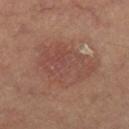The lesion was photographed on a routine skin check and not biopsied; there is no pathology result.